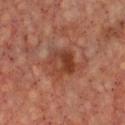workup=catalogued during a skin exam; not biopsied
patient=aged 63 to 67
location=the chest
acquisition=~15 mm crop, total-body skin-cancer survey